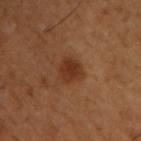{
  "biopsy_status": "not biopsied; imaged during a skin examination",
  "lesion_size": {
    "long_diameter_mm_approx": 3.0
  },
  "patient": {
    "sex": "male",
    "age_approx": 50
  },
  "site": "upper back",
  "image": {
    "source": "total-body photography crop",
    "field_of_view_mm": 15
  },
  "lighting": "cross-polarized",
  "automated_metrics": {
    "cielab_L": 34,
    "cielab_a": 23,
    "cielab_b": 32,
    "vs_skin_darker_L": 9.0,
    "vs_skin_contrast_norm": 8.0,
    "border_irregularity_0_10": 2.0,
    "color_variation_0_10": 2.5,
    "lesion_detection_confidence_0_100": 100
  }
}